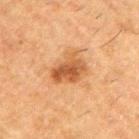{"biopsy_status": "not biopsied; imaged during a skin examination", "automated_metrics": {"area_mm2_approx": 10.0, "eccentricity": 0.7, "shape_asymmetry": 0.3, "border_irregularity_0_10": 3.5, "color_variation_0_10": 5.0, "peripheral_color_asymmetry": 1.5, "nevus_likeness_0_100": 80, "lesion_detection_confidence_0_100": 100}, "image": {"source": "total-body photography crop", "field_of_view_mm": 15}, "lesion_size": {"long_diameter_mm_approx": 4.0}, "lighting": "cross-polarized", "site": "right upper arm", "patient": {"sex": "male", "age_approx": 50}}